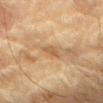Context:
A male patient aged 83 or older. On the arm. Automated image analysis of the tile measured a shape eccentricity near 0.9. And it measured a border-irregularity rating of about 3/10, a within-lesion color-variation index near 0/10, and a peripheral color-asymmetry measure near 0. It also reported an automated nevus-likeness rating near 0 out of 100 and a lesion-detection confidence of about 80/100. Approximately 2.5 mm at its widest. Cropped from a total-body skin-imaging series; the visible field is about 15 mm.